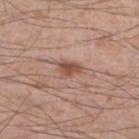Part of a total-body skin-imaging series; this lesion was reviewed on a skin check and was not flagged for biopsy. The lesion is on the right lower leg. The subject is a male in their 60s. A roughly 15 mm field-of-view crop from a total-body skin photograph. This is a white-light tile.